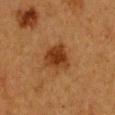notes: imaged on a skin check; not biopsied | automated lesion analysis: a lesion area of about 8 mm², a shape eccentricity near 0.45, and a shape-asymmetry score of about 0.25 (0 = symmetric); a border-irregularity rating of about 2.5/10 | location: the chest | lesion size: ~3.5 mm (longest diameter) | patient: female, aged approximately 40 | image: total-body-photography crop, ~15 mm field of view | illumination: cross-polarized illumination.